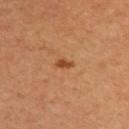Findings:
• workup · imaged on a skin check; not biopsied
• anatomic site · the upper back
• lesion size · ~2 mm (longest diameter)
• automated lesion analysis · a lesion area of about 2 mm² and an outline eccentricity of about 0.85 (0 = round, 1 = elongated); roughly 11 lightness units darker than nearby skin
• imaging modality · total-body-photography crop, ~15 mm field of view
• patient · female, aged 33–37
• tile lighting · cross-polarized illumination